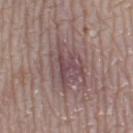Findings:
* workup — no biopsy performed (imaged during a skin exam)
* lighting — white-light
* site — the left thigh
* subject — female, about 60 years old
* size — ≈5.5 mm
* acquisition — ~15 mm crop, total-body skin-cancer survey
* image-analysis metrics — a lesion area of about 15 mm², a shape eccentricity near 0.65, and a shape-asymmetry score of about 0.35 (0 = symmetric); an average lesion color of about L≈47 a*≈17 b*≈16 (CIELAB), about 8 CIELAB-L* units darker than the surrounding skin, and a normalized lesion–skin contrast near 7; a border-irregularity rating of about 5.5/10